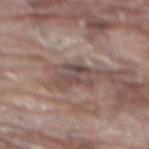Part of a total-body skin-imaging series; this lesion was reviewed on a skin check and was not flagged for biopsy. A male subject, about 80 years old. This image is a 15 mm lesion crop taken from a total-body photograph. The lesion is on the mid back. The tile uses white-light illumination. The total-body-photography lesion software estimated an average lesion color of about L≈45 a*≈15 b*≈19 (CIELAB), a lesion–skin lightness drop of about 9, and a normalized border contrast of about 7.5. The software also gave a nevus-likeness score of about 0/100 and a lesion-detection confidence of about 50/100. About 3 mm across.